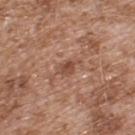Findings:
• workup · no biopsy performed (imaged during a skin exam)
• subject · male, roughly 55 years of age
• acquisition · 15 mm crop, total-body photography
• tile lighting · white-light
• automated lesion analysis · an average lesion color of about L≈49 a*≈22 b*≈29 (CIELAB) and a normalized border contrast of about 6.5; border irregularity of about 2.5 on a 0–10 scale, a color-variation rating of about 3/10, and radial color variation of about 1; a nevus-likeness score of about 0/100
• site · the upper back